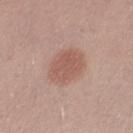Q: Was a biopsy performed?
A: imaged on a skin check; not biopsied
Q: What is the anatomic site?
A: the left thigh
Q: What did automated image analysis measure?
A: lesion-presence confidence of about 100/100
Q: Illumination type?
A: white-light illumination
Q: What kind of image is this?
A: total-body-photography crop, ~15 mm field of view
Q: What is the lesion's diameter?
A: ≈4.5 mm
Q: Patient demographics?
A: female, approximately 30 years of age